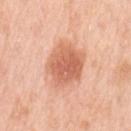No biopsy was performed on this lesion — it was imaged during a full skin examination and was not determined to be concerning. The total-body-photography lesion software estimated an area of roughly 18 mm² and an outline eccentricity of about 0.65 (0 = round, 1 = elongated). The software also gave a mean CIELAB color near L≈64 a*≈27 b*≈34, about 13 CIELAB-L* units darker than the surrounding skin, and a normalized border contrast of about 7.5. Imaged with white-light lighting. The lesion is on the left upper arm. A close-up tile cropped from a whole-body skin photograph, about 15 mm across. About 5.5 mm across. A female patient aged 48 to 52.